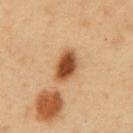The lesion was tiled from a total-body skin photograph and was not biopsied.
A male patient roughly 50 years of age.
Approximately 4 mm at its widest.
A lesion tile, about 15 mm wide, cut from a 3D total-body photograph.
This is a cross-polarized tile.
Located on the abdomen.